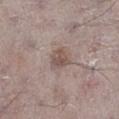Findings:
* workup — imaged on a skin check; not biopsied
* illumination — white-light
* site — the left lower leg
* lesion diameter — about 2.5 mm
* image — ~15 mm crop, total-body skin-cancer survey
* subject — male, about 70 years old